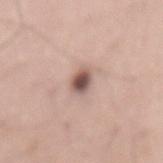Impression: Captured during whole-body skin photography for melanoma surveillance; the lesion was not biopsied. Acquisition and patient details: The lesion is located on the mid back. A 15 mm crop from a total-body photograph taken for skin-cancer surveillance. The patient is a male aged 58 to 62. This is a white-light tile.